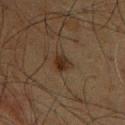biopsy_status: not biopsied; imaged during a skin examination
image:
  source: total-body photography crop
  field_of_view_mm: 15
lesion_size:
  long_diameter_mm_approx: 2.5
site: chest
lighting: cross-polarized
patient:
  sex: male
  age_approx: 60